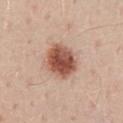<lesion>
<biopsy_status>not biopsied; imaged during a skin examination</biopsy_status>
<image>
  <source>total-body photography crop</source>
  <field_of_view_mm>15</field_of_view_mm>
</image>
<lesion_size>
  <long_diameter_mm_approx>4.5</long_diameter_mm_approx>
</lesion_size>
<lighting>white-light</lighting>
<site>lower back</site>
<patient>
  <sex>male</sex>
  <age_approx>60</age_approx>
</patient>
</lesion>